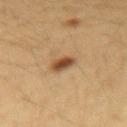Captured during whole-body skin photography for melanoma surveillance; the lesion was not biopsied. Longest diameter approximately 2.5 mm. A 15 mm close-up extracted from a 3D total-body photography capture. A female subject in their 30s. Automated image analysis of the tile measured a mean CIELAB color near L≈44 a*≈18 b*≈33, roughly 13 lightness units darker than nearby skin, and a normalized lesion–skin contrast near 10. The lesion is located on the right forearm. The tile uses cross-polarized illumination.